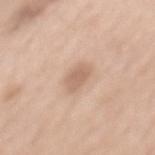Clinical summary:
A female patient roughly 65 years of age. The total-body-photography lesion software estimated a lesion area of about 4.5 mm², a shape eccentricity near 0.85, and two-axis asymmetry of about 0.25. The software also gave a lesion color around L≈63 a*≈18 b*≈29 in CIELAB, roughly 10 lightness units darker than nearby skin, and a normalized border contrast of about 6.5. It also reported a border-irregularity rating of about 2.5/10, a within-lesion color-variation index near 1.5/10, and peripheral color asymmetry of about 0.5. The lesion is on the mid back. A region of skin cropped from a whole-body photographic capture, roughly 15 mm wide. This is a white-light tile.